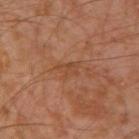{"biopsy_status": "not biopsied; imaged during a skin examination", "lesion_size": {"long_diameter_mm_approx": 2.5}, "automated_metrics": {"area_mm2_approx": 3.5, "eccentricity": 0.65, "shape_asymmetry": 0.4, "nevus_likeness_0_100": 0}, "patient": {"sex": "male", "age_approx": 30}, "image": {"source": "total-body photography crop", "field_of_view_mm": 15}, "site": "left arm", "lighting": "cross-polarized"}